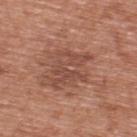This lesion was catalogued during total-body skin photography and was not selected for biopsy. A male patient, aged around 70. A region of skin cropped from a whole-body photographic capture, roughly 15 mm wide. The lesion is on the back.